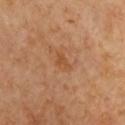Clinical impression: This lesion was catalogued during total-body skin photography and was not selected for biopsy. Clinical summary: A 15 mm close-up extracted from a 3D total-body photography capture. The tile uses cross-polarized illumination. A female patient. The lesion-visualizer software estimated a footprint of about 3.5 mm², an eccentricity of roughly 0.8, and a shape-asymmetry score of about 0.35 (0 = symmetric). The software also gave about 6 CIELAB-L* units darker than the surrounding skin and a lesion-to-skin contrast of about 5.5 (normalized; higher = more distinct). The software also gave peripheral color asymmetry of about 0.5. The analysis additionally found lesion-presence confidence of about 100/100. The lesion is on the chest. Longest diameter approximately 2.5 mm.